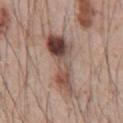Q: Was a biopsy performed?
A: imaged on a skin check; not biopsied
Q: What is the lesion's diameter?
A: about 7.5 mm
Q: Lesion location?
A: the front of the torso
Q: Patient demographics?
A: male, roughly 55 years of age
Q: How was the tile lit?
A: white-light illumination
Q: What kind of image is this?
A: ~15 mm tile from a whole-body skin photo
Q: What did automated image analysis measure?
A: an outline eccentricity of about 0.9 (0 = round, 1 = elongated) and a shape-asymmetry score of about 0.4 (0 = symmetric); about 14 CIELAB-L* units darker than the surrounding skin; a border-irregularity index near 5.5/10 and radial color variation of about 4.5; a nevus-likeness score of about 100/100 and lesion-presence confidence of about 100/100